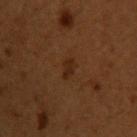biopsy status=no biopsy performed (imaged during a skin exam)
patient=male, aged 48 to 52
body site=the chest
acquisition=~15 mm tile from a whole-body skin photo
automated metrics=an average lesion color of about L≈19 a*≈15 b*≈23 (CIELAB) and a normalized border contrast of about 6; a border-irregularity index near 3/10, a color-variation rating of about 1.5/10, and a peripheral color-asymmetry measure near 0.5; a classifier nevus-likeness of about 0/100 and a lesion-detection confidence of about 100/100
illumination=cross-polarized